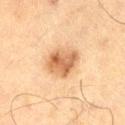Recorded during total-body skin imaging; not selected for excision or biopsy. A male subject aged around 70. About 4 mm across. The lesion-visualizer software estimated a footprint of about 11 mm², an outline eccentricity of about 0.6 (0 = round, 1 = elongated), and a shape-asymmetry score of about 0.2 (0 = symmetric). The software also gave a classifier nevus-likeness of about 90/100 and a detector confidence of about 100 out of 100 that the crop contains a lesion. From the right thigh. This image is a 15 mm lesion crop taken from a total-body photograph. The tile uses cross-polarized illumination.